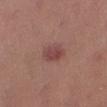Findings:
- follow-up: imaged on a skin check; not biopsied
- anatomic site: the abdomen
- patient: female, in their mid-50s
- size: ~3 mm (longest diameter)
- image source: ~15 mm tile from a whole-body skin photo
- illumination: white-light